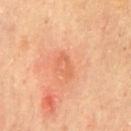Q: Was a biopsy performed?
A: catalogued during a skin exam; not biopsied
Q: What kind of image is this?
A: total-body-photography crop, ~15 mm field of view
Q: Lesion location?
A: the front of the torso
Q: What are the patient's age and sex?
A: male, approximately 65 years of age